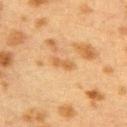Impression: This lesion was catalogued during total-body skin photography and was not selected for biopsy. Context: A 15 mm close-up tile from a total-body photography series done for melanoma screening. The subject is a female about 40 years old. About 2.5 mm across. Located on the back. The lesion-visualizer software estimated a lesion color around L≈51 a*≈19 b*≈37 in CIELAB and a lesion–skin lightness drop of about 8. The analysis additionally found an automated nevus-likeness rating near 0 out of 100 and lesion-presence confidence of about 100/100. This is a cross-polarized tile.